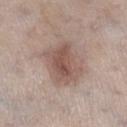Recorded during total-body skin imaging; not selected for excision or biopsy. The lesion is on the right lower leg. The subject is a male roughly 60 years of age. This is a white-light tile. A 15 mm close-up tile from a total-body photography series done for melanoma screening.